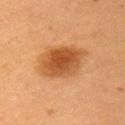Clinical impression:
Recorded during total-body skin imaging; not selected for excision or biopsy.
Image and clinical context:
A female subject, about 55 years old. Automated image analysis of the tile measured a lesion area of about 17 mm² and two-axis asymmetry of about 0.15. The software also gave a classifier nevus-likeness of about 100/100 and lesion-presence confidence of about 100/100. The lesion is on the left upper arm. A region of skin cropped from a whole-body photographic capture, roughly 15 mm wide. Measured at roughly 5 mm in maximum diameter. Imaged with cross-polarized lighting.